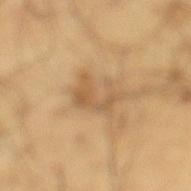lighting = cross-polarized | lesion diameter = ~4.5 mm (longest diameter) | anatomic site = the left lower leg | patient = male, in their mid-60s | image-analysis metrics = an average lesion color of about L≈58 a*≈17 b*≈38 (CIELAB), roughly 9 lightness units darker than nearby skin, and a normalized border contrast of about 6; a border-irregularity index near 8/10 and peripheral color asymmetry of about 1; a detector confidence of about 95 out of 100 that the crop contains a lesion | image source = 15 mm crop, total-body photography.